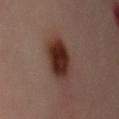notes: total-body-photography surveillance lesion; no biopsy | image-analysis metrics: an area of roughly 12 mm², an eccentricity of roughly 0.35, and two-axis asymmetry of about 0.1; a lesion color around L≈29 a*≈20 b*≈23 in CIELAB, about 14 CIELAB-L* units darker than the surrounding skin, and a normalized lesion–skin contrast near 13.5; a border-irregularity rating of about 1.5/10, internal color variation of about 4 on a 0–10 scale, and a peripheral color-asymmetry measure near 1 | size: about 4 mm | subject: female, about 60 years old | tile lighting: cross-polarized illumination | image source: 15 mm crop, total-body photography | body site: the chest.